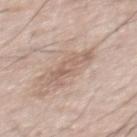notes: no biopsy performed (imaged during a skin exam)
illumination: white-light
automated lesion analysis: a lesion area of about 8 mm², an outline eccentricity of about 0.9 (0 = round, 1 = elongated), and a shape-asymmetry score of about 0.4 (0 = symmetric); a lesion color around L≈61 a*≈16 b*≈26 in CIELAB and a lesion-to-skin contrast of about 5.5 (normalized; higher = more distinct)
image source: 15 mm crop, total-body photography
diameter: ~4.5 mm (longest diameter)
subject: male, in their mid-50s
location: the upper back